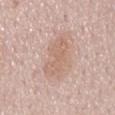| feature | finding |
|---|---|
| follow-up | imaged on a skin check; not biopsied |
| tile lighting | white-light illumination |
| site | the mid back |
| imaging modality | 15 mm crop, total-body photography |
| patient | female, about 50 years old |
| lesion size | ~5 mm (longest diameter) |
| TBP lesion metrics | an area of roughly 10 mm², a shape eccentricity near 0.9, and a shape-asymmetry score of about 0.3 (0 = symmetric) |